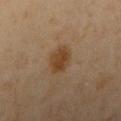Measured at roughly 3 mm in maximum diameter.
A 15 mm crop from a total-body photograph taken for skin-cancer surveillance.
A female patient, approximately 50 years of age.
On the right upper arm.
This is a cross-polarized tile.